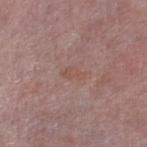* biopsy status — imaged on a skin check; not biopsied
* subject — female, in their 60s
* image — ~15 mm crop, total-body skin-cancer survey
* location — the right lower leg